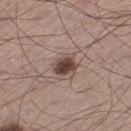The lesion was photographed on a routine skin check and not biopsied; there is no pathology result. The lesion is located on the left thigh. A male subject, in their mid- to late 50s. Imaged with white-light lighting. About 3 mm across. A 15 mm crop from a total-body photograph taken for skin-cancer surveillance.